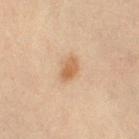Assessment:
Part of a total-body skin-imaging series; this lesion was reviewed on a skin check and was not flagged for biopsy.
Acquisition and patient details:
A 15 mm close-up extracted from a 3D total-body photography capture. From the leg. The patient is a female aged 43–47.